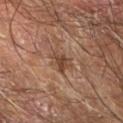{"biopsy_status": "not biopsied; imaged during a skin examination"}